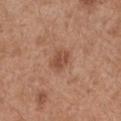follow-up — imaged on a skin check; not biopsied | image source — total-body-photography crop, ~15 mm field of view | subject — female, in their 30s | location — the right forearm.